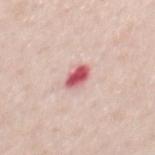Assessment: Captured during whole-body skin photography for melanoma surveillance; the lesion was not biopsied. Image and clinical context: The lesion is located on the mid back. A 15 mm close-up tile from a total-body photography series done for melanoma screening. A male subject, approximately 40 years of age. The lesion-visualizer software estimated a nevus-likeness score of about 0/100 and lesion-presence confidence of about 100/100. Longest diameter approximately 3 mm.